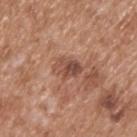- workup — catalogued during a skin exam; not biopsied
- subject — male, aged 63 to 67
- image source — 15 mm crop, total-body photography
- anatomic site — the upper back